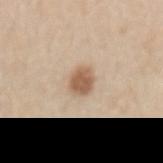The lesion was tiled from a total-body skin photograph and was not biopsied.
A region of skin cropped from a whole-body photographic capture, roughly 15 mm wide.
Imaged with white-light lighting.
A male patient roughly 60 years of age.
Located on the lower back.
About 2.5 mm across.
An algorithmic analysis of the crop reported an eccentricity of roughly 0.55 and two-axis asymmetry of about 0.2.